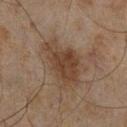notes=catalogued during a skin exam; not biopsied
imaging modality=~15 mm tile from a whole-body skin photo
lighting=cross-polarized illumination
diameter=~6 mm (longest diameter)
subject=male, aged approximately 45
body site=the right lower leg